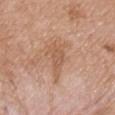biopsy status = no biopsy performed (imaged during a skin exam)
patient = male, aged approximately 75
lighting = white-light illumination
image = ~15 mm tile from a whole-body skin photo
lesion size = about 4.5 mm
site = the chest